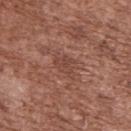notes = catalogued during a skin exam; not biopsied | anatomic site = the upper back | image = total-body-photography crop, ~15 mm field of view | patient = male, aged approximately 75 | illumination = white-light illumination.